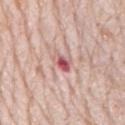<case>
<biopsy_status>not biopsied; imaged during a skin examination</biopsy_status>
<site>mid back</site>
<image>
  <source>total-body photography crop</source>
  <field_of_view_mm>15</field_of_view_mm>
</image>
<patient>
  <sex>male</sex>
  <age_approx>80</age_approx>
</patient>
</case>